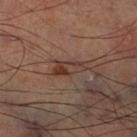The lesion was tiled from a total-body skin photograph and was not biopsied. Imaged with cross-polarized lighting. Approximately 3.5 mm at its widest. The lesion-visualizer software estimated a mean CIELAB color near L≈38 a*≈19 b*≈25, about 8 CIELAB-L* units darker than the surrounding skin, and a normalized lesion–skin contrast near 7. The analysis additionally found border irregularity of about 3 on a 0–10 scale and internal color variation of about 10 on a 0–10 scale. Located on the leg. A lesion tile, about 15 mm wide, cut from a 3D total-body photograph.